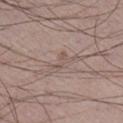<tbp_lesion>
  <biopsy_status>not biopsied; imaged during a skin examination</biopsy_status>
  <patient>
    <sex>male</sex>
    <age_approx>50</age_approx>
  </patient>
  <automated_metrics>
    <border_irregularity_0_10>5.5</border_irregularity_0_10>
    <color_variation_0_10>0.0</color_variation_0_10>
    <peripheral_color_asymmetry>0.0</peripheral_color_asymmetry>
    <nevus_likeness_0_100>0</nevus_likeness_0_100>
    <lesion_detection_confidence_0_100>75</lesion_detection_confidence_0_100>
  </automated_metrics>
  <image>
    <source>total-body photography crop</source>
    <field_of_view_mm>15</field_of_view_mm>
  </image>
  <lesion_size>
    <long_diameter_mm_approx>2.5</long_diameter_mm_approx>
  </lesion_size>
  <site>right lower leg</site>
  <lighting>white-light</lighting>
</tbp_lesion>